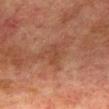follow-up=imaged on a skin check; not biopsied | acquisition=total-body-photography crop, ~15 mm field of view | lighting=cross-polarized | subject=male, in their mid-70s | lesion diameter=~3 mm (longest diameter) | site=the back.